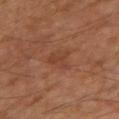Imaged during a routine full-body skin examination; the lesion was not biopsied and no histopathology is available.
Automated image analysis of the tile measured a lesion area of about 5.5 mm² and a shape-asymmetry score of about 0.45 (0 = symmetric). It also reported a lesion color around L≈40 a*≈22 b*≈30 in CIELAB, roughly 6 lightness units darker than nearby skin, and a lesion-to-skin contrast of about 5 (normalized; higher = more distinct).
Located on the arm.
A lesion tile, about 15 mm wide, cut from a 3D total-body photograph.
The patient is a male approximately 60 years of age.